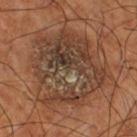Captured during whole-body skin photography for melanoma surveillance; the lesion was not biopsied.
Cropped from a total-body skin-imaging series; the visible field is about 15 mm.
The recorded lesion diameter is about 9 mm.
An algorithmic analysis of the crop reported a lesion color around L≈39 a*≈17 b*≈27 in CIELAB, a lesion–skin lightness drop of about 7, and a normalized border contrast of about 7. The analysis additionally found a within-lesion color-variation index near 9.5/10 and peripheral color asymmetry of about 3.5. The software also gave an automated nevus-likeness rating near 0 out of 100.
Located on the right thigh.
This is a cross-polarized tile.
A male subject aged 68–72.